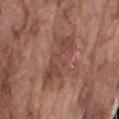Q: Was a biopsy performed?
A: no biopsy performed (imaged during a skin exam)
Q: Patient demographics?
A: male, in their mid- to late 70s
Q: Lesion size?
A: about 6.5 mm
Q: Automated lesion metrics?
A: an outline eccentricity of about 0.9 (0 = round, 1 = elongated) and two-axis asymmetry of about 0.3; an average lesion color of about L≈45 a*≈23 b*≈25 (CIELAB) and roughly 7 lightness units darker than nearby skin; a color-variation rating of about 4/10
Q: What kind of image is this?
A: ~15 mm crop, total-body skin-cancer survey
Q: Where on the body is the lesion?
A: the arm
Q: What lighting was used for the tile?
A: white-light